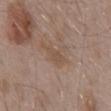<record>
  <biopsy_status>not biopsied; imaged during a skin examination</biopsy_status>
  <lighting>white-light</lighting>
  <patient>
    <sex>male</sex>
    <age_approx>55</age_approx>
  </patient>
  <site>back</site>
  <image>
    <source>total-body photography crop</source>
    <field_of_view_mm>15</field_of_view_mm>
  </image>
  <automated_metrics>
    <area_mm2_approx>3.5</area_mm2_approx>
    <eccentricity>0.9</eccentricity>
    <shape_asymmetry>0.3</shape_asymmetry>
    <lesion_detection_confidence_0_100>100</lesion_detection_confidence_0_100>
  </automated_metrics>
</record>